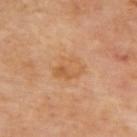biopsy status = total-body-photography surveillance lesion; no biopsy | diameter = ≈3.5 mm | site = the upper back | image = total-body-photography crop, ~15 mm field of view | lighting = cross-polarized illumination.